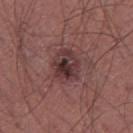Acquisition and patient details:
A 15 mm crop from a total-body photograph taken for skin-cancer surveillance. A male patient, about 45 years old. The lesion is located on the right thigh. The tile uses white-light illumination. Measured at roughly 4 mm in maximum diameter.